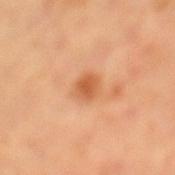biopsy_status: not biopsied; imaged during a skin examination
patient:
  sex: female
  age_approx: 35
site: left lower leg
lighting: cross-polarized
image:
  source: total-body photography crop
  field_of_view_mm: 15